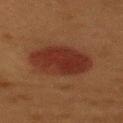Clinical impression:
This lesion was catalogued during total-body skin photography and was not selected for biopsy.
Background:
A region of skin cropped from a whole-body photographic capture, roughly 15 mm wide. A female patient, aged approximately 50. On the back.